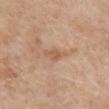workup — catalogued during a skin exam; not biopsied
body site — the mid back
image-analysis metrics — a footprint of about 3.5 mm², an outline eccentricity of about 0.9 (0 = round, 1 = elongated), and a shape-asymmetry score of about 0.3 (0 = symmetric); a lesion-to-skin contrast of about 6 (normalized; higher = more distinct); internal color variation of about 1 on a 0–10 scale and peripheral color asymmetry of about 0.5; a detector confidence of about 100 out of 100 that the crop contains a lesion
image source — 15 mm crop, total-body photography
patient — female, roughly 60 years of age
size — ~3 mm (longest diameter)
lighting — white-light